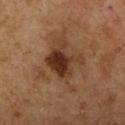workup=no biopsy performed (imaged during a skin exam) | lesion size=about 8 mm | location=the left upper arm | image=total-body-photography crop, ~15 mm field of view | subject=female, approximately 60 years of age.